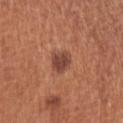Recorded during total-body skin imaging; not selected for excision or biopsy. Captured under white-light illumination. Approximately 3.5 mm at its widest. A 15 mm close-up extracted from a 3D total-body photography capture. A female subject, in their mid-60s. The lesion is located on the left upper arm.